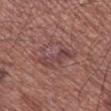Clinical impression: Recorded during total-body skin imaging; not selected for excision or biopsy. Background: A male subject approximately 65 years of age. Located on the right lower leg. A region of skin cropped from a whole-body photographic capture, roughly 15 mm wide. About 4.5 mm across.